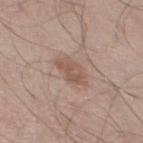Clinical impression:
Captured during whole-body skin photography for melanoma surveillance; the lesion was not biopsied.
Context:
Imaged with white-light lighting. On the upper back. An algorithmic analysis of the crop reported a lesion color around L≈54 a*≈18 b*≈26 in CIELAB and a lesion–skin lightness drop of about 8. Measured at roughly 3.5 mm in maximum diameter. A male patient, in their mid- to late 40s. A 15 mm crop from a total-body photograph taken for skin-cancer surveillance.